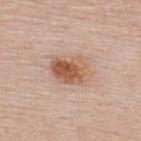  biopsy_status: not biopsied; imaged during a skin examination
  patient:
    sex: female
    age_approx: 50
  image:
    source: total-body photography crop
    field_of_view_mm: 15
  lighting: white-light
  lesion_size:
    long_diameter_mm_approx: 5.0
  automated_metrics:
    area_mm2_approx: 13.0
    eccentricity: 0.7
    shape_asymmetry: 0.2
    nevus_likeness_0_100: 95
  site: upper back